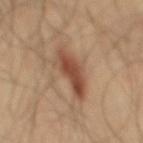Imaged during a routine full-body skin examination; the lesion was not biopsied and no histopathology is available.
Approximately 6 mm at its widest.
A 15 mm close-up tile from a total-body photography series done for melanoma screening.
The tile uses cross-polarized illumination.
The subject is a male aged 63–67.
The lesion is on the mid back.